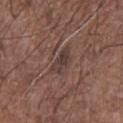{"image": {"source": "total-body photography crop", "field_of_view_mm": 15}, "patient": {"sex": "male", "age_approx": 70}, "site": "chest"}